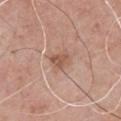The lesion was photographed on a routine skin check and not biopsied; there is no pathology result. On the front of the torso. The total-body-photography lesion software estimated a mean CIELAB color near L≈55 a*≈20 b*≈29 and a lesion–skin lightness drop of about 9. Captured under white-light illumination. A male subject, about 60 years old. Cropped from a whole-body photographic skin survey; the tile spans about 15 mm.